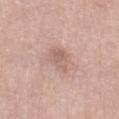Q: Was this lesion biopsied?
A: total-body-photography surveillance lesion; no biopsy
Q: Who is the patient?
A: female, roughly 65 years of age
Q: What lighting was used for the tile?
A: white-light
Q: Lesion location?
A: the right thigh
Q: What is the lesion's diameter?
A: ~3.5 mm (longest diameter)
Q: What is the imaging modality?
A: ~15 mm crop, total-body skin-cancer survey
Q: What did automated image analysis measure?
A: a shape eccentricity near 0.75 and a symmetry-axis asymmetry near 0.25; a border-irregularity rating of about 2.5/10, a within-lesion color-variation index near 3/10, and radial color variation of about 1; a detector confidence of about 100 out of 100 that the crop contains a lesion